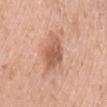The lesion was tiled from a total-body skin photograph and was not biopsied. The patient is a female aged around 65. The total-body-photography lesion software estimated an area of roughly 11 mm² and a shape-asymmetry score of about 0.25 (0 = symmetric). The analysis additionally found a mean CIELAB color near L≈59 a*≈23 b*≈31, a lesion–skin lightness drop of about 12, and a lesion-to-skin contrast of about 7.5 (normalized; higher = more distinct). On the left upper arm. A close-up tile cropped from a whole-body skin photograph, about 15 mm across. The lesion's longest dimension is about 4.5 mm.